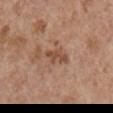The lesion was photographed on a routine skin check and not biopsied; there is no pathology result. From the right upper arm. Captured under white-light illumination. Cropped from a total-body skin-imaging series; the visible field is about 15 mm. The subject is a female aged approximately 65. The lesion's longest dimension is about 3 mm.